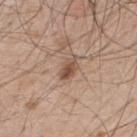Notes:
– follow-up: catalogued during a skin exam; not biopsied
– location: the upper back
– diameter: about 3.5 mm
– subject: male, aged 48–52
– acquisition: 15 mm crop, total-body photography